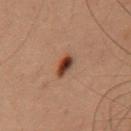Q: How large is the lesion?
A: ~3 mm (longest diameter)
Q: Illumination type?
A: cross-polarized illumination
Q: What is the imaging modality?
A: ~15 mm crop, total-body skin-cancer survey
Q: Patient demographics?
A: male, in their 60s
Q: Where on the body is the lesion?
A: the chest